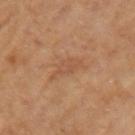location: the arm | image source: total-body-photography crop, ~15 mm field of view | size: ≈4 mm | illumination: cross-polarized illumination | patient: female, aged approximately 65 | automated metrics: an area of roughly 5.5 mm², an eccentricity of roughly 0.9, and a shape-asymmetry score of about 0.4 (0 = symmetric); a mean CIELAB color near L≈50 a*≈21 b*≈32 and about 6 CIELAB-L* units darker than the surrounding skin; a border-irregularity rating of about 4.5/10, a color-variation rating of about 1.5/10, and a peripheral color-asymmetry measure near 0.5; an automated nevus-likeness rating near 0 out of 100 and a lesion-detection confidence of about 100/100.